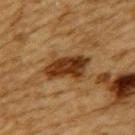subject = male, in their mid-80s or older | imaging modality = 15 mm crop, total-body photography | location = the upper back.